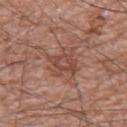This is a white-light tile.
This image is a 15 mm lesion crop taken from a total-body photograph.
The recorded lesion diameter is about 3.5 mm.
The total-body-photography lesion software estimated roughly 8 lightness units darker than nearby skin. The software also gave border irregularity of about 4.5 on a 0–10 scale, a within-lesion color-variation index near 3.5/10, and peripheral color asymmetry of about 1.
The subject is a male aged approximately 60.
The lesion is located on the leg.